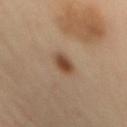Imaged during a routine full-body skin examination; the lesion was not biopsied and no histopathology is available.
On the mid back.
About 3 mm across.
The tile uses cross-polarized illumination.
A male patient, aged approximately 55.
A region of skin cropped from a whole-body photographic capture, roughly 15 mm wide.